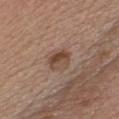A roughly 15 mm field-of-view crop from a total-body skin photograph. Located on the chest. Longest diameter approximately 3 mm. A male subject about 55 years old.